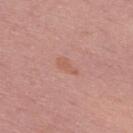Impression: Part of a total-body skin-imaging series; this lesion was reviewed on a skin check and was not flagged for biopsy. Acquisition and patient details: Captured under white-light illumination. The lesion-visualizer software estimated an area of roughly 3 mm², an outline eccentricity of about 0.85 (0 = round, 1 = elongated), and a shape-asymmetry score of about 0.35 (0 = symmetric). A male patient, roughly 55 years of age. A roughly 15 mm field-of-view crop from a total-body skin photograph. From the upper back.